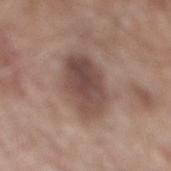Case summary:
• biopsy status: imaged on a skin check; not biopsied
• patient: male, aged 58–62
• image: ~15 mm crop, total-body skin-cancer survey
• body site: the mid back